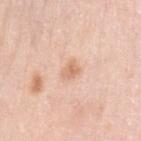Impression:
Imaged during a routine full-body skin examination; the lesion was not biopsied and no histopathology is available.
Context:
The recorded lesion diameter is about 2.5 mm. Captured under white-light illumination. A female subject in their mid-60s. The lesion is on the left upper arm. A 15 mm crop from a total-body photograph taken for skin-cancer surveillance.